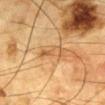Q: Is there a histopathology result?
A: catalogued during a skin exam; not biopsied
Q: How large is the lesion?
A: ~4 mm (longest diameter)
Q: Automated lesion metrics?
A: a lesion–skin lightness drop of about 8
Q: How was this image acquired?
A: 15 mm crop, total-body photography
Q: Lesion location?
A: the chest
Q: Who is the patient?
A: male, about 85 years old or older
Q: How was the tile lit?
A: cross-polarized